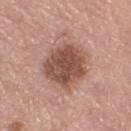Q: Was this lesion biopsied?
A: total-body-photography surveillance lesion; no biopsy
Q: What are the patient's age and sex?
A: male, aged 68–72
Q: Lesion size?
A: about 5.5 mm
Q: What is the anatomic site?
A: the right thigh
Q: What did automated image analysis measure?
A: an area of roughly 19 mm², an outline eccentricity of about 0.45 (0 = round, 1 = elongated), and two-axis asymmetry of about 0.15; an average lesion color of about L≈50 a*≈22 b*≈25 (CIELAB), a lesion–skin lightness drop of about 14, and a normalized border contrast of about 9.5; an automated nevus-likeness rating near 40 out of 100 and lesion-presence confidence of about 100/100
Q: What is the imaging modality?
A: ~15 mm tile from a whole-body skin photo